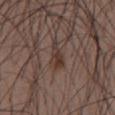biopsy status: total-body-photography surveillance lesion; no biopsy
illumination: white-light
lesion size: ~3.5 mm (longest diameter)
patient: male, aged 38 to 42
location: the chest
image: ~15 mm tile from a whole-body skin photo
TBP lesion metrics: a footprint of about 4.5 mm², a shape eccentricity near 0.85, and a shape-asymmetry score of about 0.45 (0 = symmetric); a lesion color around L≈35 a*≈16 b*≈22 in CIELAB and a lesion–skin lightness drop of about 8; a border-irregularity rating of about 5/10, internal color variation of about 3 on a 0–10 scale, and radial color variation of about 1; a nevus-likeness score of about 55/100 and a detector confidence of about 95 out of 100 that the crop contains a lesion